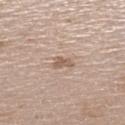Clinical impression: No biopsy was performed on this lesion — it was imaged during a full skin examination and was not determined to be concerning. Background: A 15 mm close-up extracted from a 3D total-body photography capture. Automated tile analysis of the lesion measured a shape-asymmetry score of about 0.45 (0 = symmetric). It also reported an average lesion color of about L≈58 a*≈17 b*≈27 (CIELAB), about 10 CIELAB-L* units darker than the surrounding skin, and a normalized border contrast of about 6.5. It also reported a border-irregularity index near 4/10, a color-variation rating of about 1/10, and peripheral color asymmetry of about 0.5. A female patient, approximately 70 years of age. The tile uses white-light illumination. On the left lower leg.